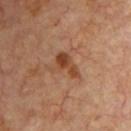The lesion was photographed on a routine skin check and not biopsied; there is no pathology result. The lesion is on the chest. The lesion-visualizer software estimated an automated nevus-likeness rating near 10 out of 100 and a detector confidence of about 100 out of 100 that the crop contains a lesion. Cropped from a total-body skin-imaging series; the visible field is about 15 mm. A subject in their mid- to late 60s. About 3.5 mm across. Captured under cross-polarized illumination.